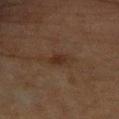patient:
  sex: female
  age_approx: 65
site: arm
automated_metrics:
  area_mm2_approx: 4.5
  shape_asymmetry: 0.25
image:
  source: total-body photography crop
  field_of_view_mm: 15
lesion_size:
  long_diameter_mm_approx: 3.5